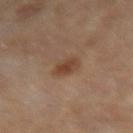Captured during whole-body skin photography for melanoma surveillance; the lesion was not biopsied.
This is a cross-polarized tile.
Longest diameter approximately 2.5 mm.
A female subject, in their 50s.
From the left thigh.
The lesion-visualizer software estimated a lesion area of about 5 mm², an outline eccentricity of about 0.4 (0 = round, 1 = elongated), and a shape-asymmetry score of about 0.2 (0 = symmetric). It also reported an average lesion color of about L≈41 a*≈18 b*≈28 (CIELAB), a lesion–skin lightness drop of about 9, and a normalized border contrast of about 7.5. And it measured a nevus-likeness score of about 80/100.
A region of skin cropped from a whole-body photographic capture, roughly 15 mm wide.